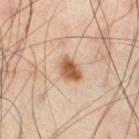  biopsy_status: not biopsied; imaged during a skin examination
  patient:
    sex: male
    age_approx: 55
  image:
    source: total-body photography crop
    field_of_view_mm: 15
  site: right thigh